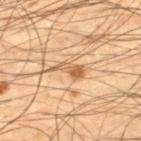  biopsy_status: not biopsied; imaged during a skin examination
  patient:
    sex: male
    age_approx: 45
  lighting: cross-polarized
  lesion_size:
    long_diameter_mm_approx: 4.0
  site: right lower leg
  image:
    source: total-body photography crop
    field_of_view_mm: 15